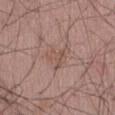workup = imaged on a skin check; not biopsied | tile lighting = white-light | anatomic site = the abdomen | acquisition = total-body-photography crop, ~15 mm field of view | patient = male, roughly 25 years of age | size = about 3.5 mm | automated metrics = roughly 6 lightness units darker than nearby skin.